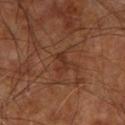Captured during whole-body skin photography for melanoma surveillance; the lesion was not biopsied. Measured at roughly 2.5 mm in maximum diameter. On the right upper arm. A roughly 15 mm field-of-view crop from a total-body skin photograph. The patient is roughly 65 years of age. Captured under cross-polarized illumination. Automated image analysis of the tile measured a lesion color around L≈32 a*≈22 b*≈28 in CIELAB, about 6 CIELAB-L* units darker than the surrounding skin, and a normalized lesion–skin contrast near 5.5. The analysis additionally found a border-irregularity rating of about 5.5/10, a within-lesion color-variation index near 0/10, and a peripheral color-asymmetry measure near 0.